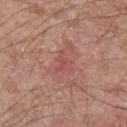Captured during whole-body skin photography for melanoma surveillance; the lesion was not biopsied.
A male subject, approximately 65 years of age.
Measured at roughly 3.5 mm in maximum diameter.
A lesion tile, about 15 mm wide, cut from a 3D total-body photograph.
Located on the left forearm.
Imaged with white-light lighting.
An algorithmic analysis of the crop reported a color-variation rating of about 2/10 and a peripheral color-asymmetry measure near 0.5. It also reported an automated nevus-likeness rating near 0 out of 100 and a lesion-detection confidence of about 95/100.